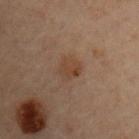| feature | finding |
|---|---|
| workup | catalogued during a skin exam; not biopsied |
| anatomic site | the chest |
| imaging modality | ~15 mm tile from a whole-body skin photo |
| tile lighting | cross-polarized |
| subject | male, in their mid- to late 50s |
| size | ≈2.5 mm |